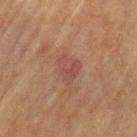workup: catalogued during a skin exam; not biopsied
subject: female, aged approximately 80
site: the arm
acquisition: 15 mm crop, total-body photography